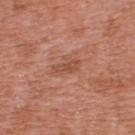Assessment: The lesion was tiled from a total-body skin photograph and was not biopsied. Clinical summary: A male subject about 70 years old. A 15 mm close-up tile from a total-body photography series done for melanoma screening. On the upper back.